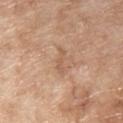Clinical impression:
No biopsy was performed on this lesion — it was imaged during a full skin examination and was not determined to be concerning.
Clinical summary:
About 3 mm across. Captured under white-light illumination. Cropped from a whole-body photographic skin survey; the tile spans about 15 mm. Located on the chest. A male subject, aged 63 to 67.